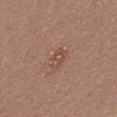The lesion was tiled from a total-body skin photograph and was not biopsied.
A male patient about 55 years old.
A region of skin cropped from a whole-body photographic capture, roughly 15 mm wide.
Automated tile analysis of the lesion measured a lesion area of about 3.5 mm², an outline eccentricity of about 0.8 (0 = round, 1 = elongated), and a symmetry-axis asymmetry near 0.25. And it measured an average lesion color of about L≈49 a*≈20 b*≈27 (CIELAB), roughly 6 lightness units darker than nearby skin, and a normalized border contrast of about 5. The software also gave a within-lesion color-variation index near 3/10 and radial color variation of about 1.5. The analysis additionally found lesion-presence confidence of about 100/100.
The lesion is located on the upper back.
Longest diameter approximately 2.5 mm.